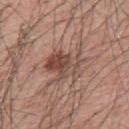workup — catalogued during a skin exam; not biopsied | location — the mid back | lighting — white-light illumination | image source — 15 mm crop, total-body photography | patient — male, in their mid- to late 50s | size — ~5.5 mm (longest diameter).